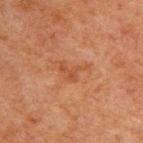| key | value |
|---|---|
| tile lighting | cross-polarized illumination |
| site | the back |
| diameter | ≈3.5 mm |
| subject | male, aged approximately 60 |
| image source | ~15 mm tile from a whole-body skin photo |